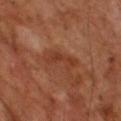<record>
<patient>
  <sex>male</sex>
  <age_approx>65</age_approx>
</patient>
<lesion_size>
  <long_diameter_mm_approx>5.0</long_diameter_mm_approx>
</lesion_size>
<lighting>cross-polarized</lighting>
<automated_metrics>
  <eccentricity>0.95</eccentricity>
  <cielab_L>38</cielab_L>
  <cielab_a>25</cielab_a>
  <cielab_b>32</cielab_b>
  <vs_skin_darker_L>7.0</vs_skin_darker_L>
  <border_irregularity_0_10>5.0</border_irregularity_0_10>
  <color_variation_0_10>2.5</color_variation_0_10>
  <nevus_likeness_0_100>0</nevus_likeness_0_100>
  <lesion_detection_confidence_0_100>100</lesion_detection_confidence_0_100>
</automated_metrics>
<image>
  <source>total-body photography crop</source>
  <field_of_view_mm>15</field_of_view_mm>
</image>
</record>